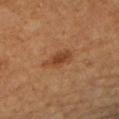– biopsy status: no biopsy performed (imaged during a skin exam)
– subject: female, aged 58 to 62
– imaging modality: 15 mm crop, total-body photography
– illumination: cross-polarized
– anatomic site: the left forearm
– lesion diameter: ≈4 mm